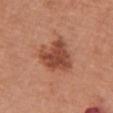Q: Was this lesion biopsied?
A: total-body-photography surveillance lesion; no biopsy
Q: How was the tile lit?
A: white-light illumination
Q: Automated lesion metrics?
A: an area of roughly 14 mm², a shape eccentricity near 0.4, and two-axis asymmetry of about 0.3; a lesion color around L≈48 a*≈26 b*≈32 in CIELAB, about 12 CIELAB-L* units darker than the surrounding skin, and a lesion-to-skin contrast of about 8.5 (normalized; higher = more distinct); border irregularity of about 3.5 on a 0–10 scale, a color-variation rating of about 3.5/10, and peripheral color asymmetry of about 1
Q: Who is the patient?
A: female, aged 63–67
Q: What is the anatomic site?
A: the chest
Q: What is the lesion's diameter?
A: ~4.5 mm (longest diameter)
Q: What kind of image is this?
A: ~15 mm crop, total-body skin-cancer survey